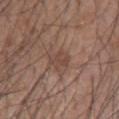{
  "biopsy_status": "not biopsied; imaged during a skin examination",
  "site": "left upper arm",
  "image": {
    "source": "total-body photography crop",
    "field_of_view_mm": 15
  },
  "patient": {
    "sex": "male",
    "age_approx": 55
  }
}